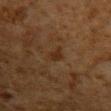{
  "image": {
    "source": "total-body photography crop",
    "field_of_view_mm": 15
  },
  "patient": {
    "sex": "male",
    "age_approx": 60
  },
  "lesion_size": {
    "long_diameter_mm_approx": 2.5
  },
  "site": "left upper arm"
}